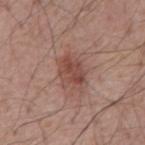Imaged during a routine full-body skin examination; the lesion was not biopsied and no histopathology is available. From the mid back. The patient is a male aged around 55. Captured under white-light illumination. The lesion's longest dimension is about 4.5 mm. A 15 mm close-up tile from a total-body photography series done for melanoma screening.